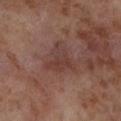Findings:
- notes: total-body-photography surveillance lesion; no biopsy
- subject: female, aged approximately 55
- imaging modality: total-body-photography crop, ~15 mm field of view
- body site: the leg